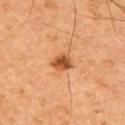notes — total-body-photography surveillance lesion; no biopsy
TBP lesion metrics — a border-irregularity rating of about 2/10, a within-lesion color-variation index near 3/10, and radial color variation of about 1; a nevus-likeness score of about 95/100
patient — male, in their mid-60s
image — ~15 mm tile from a whole-body skin photo
lighting — cross-polarized
site — the left upper arm
lesion size — about 2.5 mm